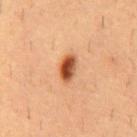biopsy status = catalogued during a skin exam; not biopsied | subject = male, aged around 55 | tile lighting = cross-polarized illumination | automated lesion analysis = border irregularity of about 2 on a 0–10 scale, a within-lesion color-variation index near 8.5/10, and peripheral color asymmetry of about 3; a classifier nevus-likeness of about 100/100 | acquisition = 15 mm crop, total-body photography | site = the mid back.